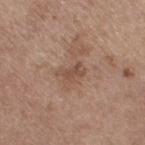  biopsy_status: not biopsied; imaged during a skin examination
  site: right thigh
  lighting: white-light
  automated_metrics:
    area_mm2_approx: 3.5
    eccentricity: 0.85
    shape_asymmetry: 0.4
    cielab_L: 49
    cielab_a: 19
    cielab_b: 27
    vs_skin_darker_L: 9.0
    vs_skin_contrast_norm: 6.5
    border_irregularity_0_10: 4.5
    peripheral_color_asymmetry: 0.0
    nevus_likeness_0_100: 0
    lesion_detection_confidence_0_100: 100
  image:
    source: total-body photography crop
    field_of_view_mm: 15
  lesion_size:
    long_diameter_mm_approx: 3.0
  patient:
    sex: female
    age_approx: 65